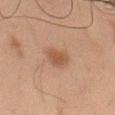notes: imaged on a skin check; not biopsied | anatomic site: the left thigh | imaging modality: ~15 mm crop, total-body skin-cancer survey | subject: male, approximately 45 years of age.